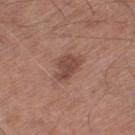{
  "biopsy_status": "not biopsied; imaged during a skin examination",
  "patient": {
    "sex": "male",
    "age_approx": 65
  },
  "site": "leg",
  "lesion_size": {
    "long_diameter_mm_approx": 4.0
  },
  "image": {
    "source": "total-body photography crop",
    "field_of_view_mm": 15
  },
  "automated_metrics": {
    "cielab_L": 46,
    "cielab_a": 21,
    "cielab_b": 26,
    "vs_skin_darker_L": 10.0
  }
}